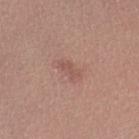biopsy_status: not biopsied; imaged during a skin examination
image:
  source: total-body photography crop
  field_of_view_mm: 15
site: left thigh
lighting: white-light
automated_metrics:
  cielab_L: 53
  cielab_a: 22
  cielab_b: 25
  vs_skin_darker_L: 7.0
  vs_skin_contrast_norm: 5.0
  border_irregularity_0_10: 4.0
  color_variation_0_10: 0.0
  peripheral_color_asymmetry: 0.0
lesion_size:
  long_diameter_mm_approx: 2.5
patient:
  sex: female
  age_approx: 25